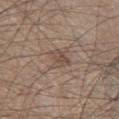This lesion was catalogued during total-body skin photography and was not selected for biopsy.
Longest diameter approximately 2.5 mm.
A male patient, about 45 years old.
Automated tile analysis of the lesion measured a lesion area of about 5 mm², an eccentricity of roughly 0.6, and a symmetry-axis asymmetry near 0.4. The analysis additionally found a lesion color around L≈48 a*≈15 b*≈24 in CIELAB, about 7 CIELAB-L* units darker than the surrounding skin, and a normalized lesion–skin contrast near 5.5. And it measured an automated nevus-likeness rating near 0 out of 100 and a lesion-detection confidence of about 95/100.
This is a white-light tile.
Located on the left thigh.
A lesion tile, about 15 mm wide, cut from a 3D total-body photograph.